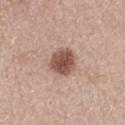The lesion was photographed on a routine skin check and not biopsied; there is no pathology result. A close-up tile cropped from a whole-body skin photograph, about 15 mm across. The lesion's longest dimension is about 3.5 mm. The lesion is on the left forearm. A female subject aged 48–52.